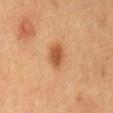Findings:
– follow-up — no biopsy performed (imaged during a skin exam)
– patient — male, roughly 60 years of age
– acquisition — ~15 mm crop, total-body skin-cancer survey
– lighting — cross-polarized illumination
– location — the abdomen
– size — ~3.5 mm (longest diameter)
– automated lesion analysis — an average lesion color of about L≈49 a*≈23 b*≈37 (CIELAB), roughly 11 lightness units darker than nearby skin, and a normalized lesion–skin contrast near 8.5; a color-variation rating of about 3.5/10